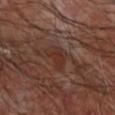<record>
<biopsy_status>not biopsied; imaged during a skin examination</biopsy_status>
<lighting>cross-polarized</lighting>
<lesion_size>
  <long_diameter_mm_approx>3.0</long_diameter_mm_approx>
</lesion_size>
<automated_metrics>
  <eccentricity>0.65</eccentricity>
  <shape_asymmetry>0.45</shape_asymmetry>
  <cielab_L>30</cielab_L>
  <cielab_a>20</cielab_a>
  <cielab_b>23</cielab_b>
  <vs_skin_darker_L>6.0</vs_skin_darker_L>
  <nevus_likeness_0_100>0</nevus_likeness_0_100>
</automated_metrics>
<image>
  <source>total-body photography crop</source>
  <field_of_view_mm>15</field_of_view_mm>
</image>
<site>right forearm</site>
<patient>
  <sex>male</sex>
  <age_approx>65</age_approx>
</patient>
</record>